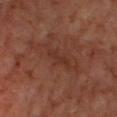Impression:
Imaged during a routine full-body skin examination; the lesion was not biopsied and no histopathology is available.
Background:
A roughly 15 mm field-of-view crop from a total-body skin photograph. From the right thigh. Captured under cross-polarized illumination. The subject is a male in their 60s. Longest diameter approximately 3 mm.